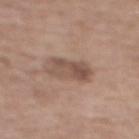  biopsy_status: not biopsied; imaged during a skin examination
  patient:
    sex: female
    age_approx: 65
  lighting: white-light
  image:
    source: total-body photography crop
    field_of_view_mm: 15
  site: mid back